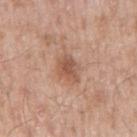Q: Was this lesion biopsied?
A: total-body-photography surveillance lesion; no biopsy
Q: What did automated image analysis measure?
A: border irregularity of about 2 on a 0–10 scale and peripheral color asymmetry of about 1; a classifier nevus-likeness of about 65/100
Q: How was the tile lit?
A: white-light illumination
Q: How was this image acquired?
A: ~15 mm crop, total-body skin-cancer survey
Q: Who is the patient?
A: male, aged 53 to 57
Q: Where on the body is the lesion?
A: the mid back